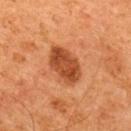notes: total-body-photography surveillance lesion; no biopsy | tile lighting: cross-polarized | image: ~15 mm tile from a whole-body skin photo | patient: male, roughly 65 years of age | lesion diameter: about 5 mm | location: the upper back.